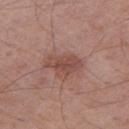Impression:
The lesion was photographed on a routine skin check and not biopsied; there is no pathology result.
Context:
The tile uses white-light illumination. The lesion's longest dimension is about 4.5 mm. The patient is a male aged around 55. The lesion is located on the leg. A 15 mm crop from a total-body photograph taken for skin-cancer surveillance.